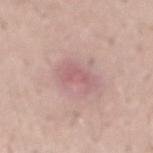– follow-up: total-body-photography surveillance lesion; no biopsy
– automated metrics: a mean CIELAB color near L≈60 a*≈23 b*≈20 and a lesion–skin lightness drop of about 8; a border-irregularity index near 4/10, internal color variation of about 2.5 on a 0–10 scale, and radial color variation of about 1
– illumination: white-light
– site: the lower back
– patient: male, approximately 40 years of age
– diameter: ≈3.5 mm
– imaging modality: ~15 mm tile from a whole-body skin photo